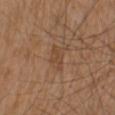Part of a total-body skin-imaging series; this lesion was reviewed on a skin check and was not flagged for biopsy.
A 15 mm crop from a total-body photograph taken for skin-cancer surveillance.
Approximately 3 mm at its widest.
A male patient, in their mid-70s.
On the back.
Captured under white-light illumination.
The lesion-visualizer software estimated a lesion color around L≈46 a*≈19 b*≈31 in CIELAB, about 6 CIELAB-L* units darker than the surrounding skin, and a normalized border contrast of about 5. The software also gave border irregularity of about 3.5 on a 0–10 scale, internal color variation of about 2.5 on a 0–10 scale, and radial color variation of about 1. It also reported a nevus-likeness score of about 0/100 and a detector confidence of about 100 out of 100 that the crop contains a lesion.